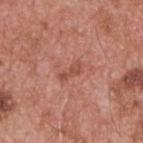notes: total-body-photography surveillance lesion; no biopsy | patient: male, aged 53–57 | lesion size: ≈3 mm | image: total-body-photography crop, ~15 mm field of view | location: the upper back | tile lighting: white-light.